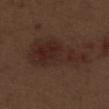follow-up: imaged on a skin check; not biopsied